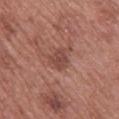Case summary:
– notes · catalogued during a skin exam; not biopsied
– image · total-body-photography crop, ~15 mm field of view
– diameter · about 3 mm
– subject · female, aged 58–62
– lighting · white-light illumination
– location · the upper back
– automated lesion analysis · a footprint of about 5.5 mm², a shape eccentricity near 0.55, and two-axis asymmetry of about 0.35; a mean CIELAB color near L≈46 a*≈23 b*≈25, a lesion–skin lightness drop of about 9, and a normalized border contrast of about 6.5; border irregularity of about 3 on a 0–10 scale, internal color variation of about 2 on a 0–10 scale, and peripheral color asymmetry of about 0.5; a classifier nevus-likeness of about 15/100